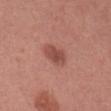Assessment: Part of a total-body skin-imaging series; this lesion was reviewed on a skin check and was not flagged for biopsy. Clinical summary: A region of skin cropped from a whole-body photographic capture, roughly 15 mm wide. On the head or neck. The patient is a female aged approximately 25.